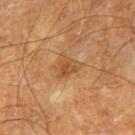Automated tile analysis of the lesion measured an area of roughly 5 mm² and a shape eccentricity near 0.7. It also reported an average lesion color of about L≈49 a*≈21 b*≈37 (CIELAB), about 8 CIELAB-L* units darker than the surrounding skin, and a lesion-to-skin contrast of about 6 (normalized; higher = more distinct).
The patient is a male approximately 65 years of age.
Captured under cross-polarized illumination.
Located on the left thigh.
The lesion's longest dimension is about 3 mm.
Cropped from a total-body skin-imaging series; the visible field is about 15 mm.